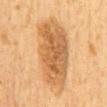Clinical impression: Captured during whole-body skin photography for melanoma surveillance; the lesion was not biopsied. Clinical summary: From the back. A male patient aged approximately 60. A 15 mm close-up tile from a total-body photography series done for melanoma screening. Captured under cross-polarized illumination. The recorded lesion diameter is about 10 mm.